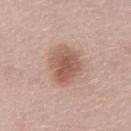follow-up: total-body-photography surveillance lesion; no biopsy | automated metrics: a mean CIELAB color near L≈57 a*≈20 b*≈27, roughly 12 lightness units darker than nearby skin, and a normalized lesion–skin contrast near 8; a detector confidence of about 100 out of 100 that the crop contains a lesion | location: the abdomen | lighting: white-light illumination | subject: male, aged 63–67 | image: total-body-photography crop, ~15 mm field of view | diameter: about 5 mm.